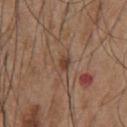Acquisition and patient details:
Cropped from a total-body skin-imaging series; the visible field is about 15 mm. Captured under white-light illumination. On the chest. Automated tile analysis of the lesion measured a lesion area of about 3.5 mm², a shape eccentricity near 0.85, and a shape-asymmetry score of about 0.3 (0 = symmetric). The analysis additionally found a lesion color around L≈43 a*≈18 b*≈28 in CIELAB, about 8 CIELAB-L* units darker than the surrounding skin, and a lesion-to-skin contrast of about 6.5 (normalized; higher = more distinct). And it measured a border-irregularity index near 2.5/10 and a within-lesion color-variation index near 3/10. It also reported a nevus-likeness score of about 0/100. The subject is a male in their mid- to late 50s. About 3 mm across.